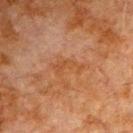Clinical impression:
No biopsy was performed on this lesion — it was imaged during a full skin examination and was not determined to be concerning.
Clinical summary:
Automated tile analysis of the lesion measured an area of roughly 5 mm², an outline eccentricity of about 0.85 (0 = round, 1 = elongated), and two-axis asymmetry of about 0.5. The analysis additionally found a border-irregularity index near 7/10 and internal color variation of about 1 on a 0–10 scale. It also reported a classifier nevus-likeness of about 0/100. On the chest. The recorded lesion diameter is about 4 mm. A male subject in their 80s. A close-up tile cropped from a whole-body skin photograph, about 15 mm across.